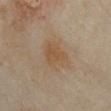The lesion was photographed on a routine skin check and not biopsied; there is no pathology result.
About 4.5 mm across.
Captured under cross-polarized illumination.
A female subject, aged around 35.
Automated tile analysis of the lesion measured an average lesion color of about L≈49 a*≈15 b*≈30 (CIELAB), about 6 CIELAB-L* units darker than the surrounding skin, and a normalized lesion–skin contrast near 6. The analysis additionally found border irregularity of about 3 on a 0–10 scale, internal color variation of about 2.5 on a 0–10 scale, and peripheral color asymmetry of about 0.5. The software also gave an automated nevus-likeness rating near 10 out of 100 and a lesion-detection confidence of about 100/100.
A 15 mm close-up tile from a total-body photography series done for melanoma screening.
The lesion is on the chest.